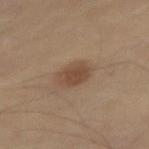Acquisition and patient details: The lesion is located on the right thigh. A roughly 15 mm field-of-view crop from a total-body skin photograph. The subject is a male aged around 55. This is a cross-polarized tile.